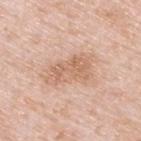Recorded during total-body skin imaging; not selected for excision or biopsy. A region of skin cropped from a whole-body photographic capture, roughly 15 mm wide. The patient is a male aged 48 to 52. Located on the upper back. About 6 mm across.